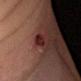biopsy_status: not biopsied; imaged during a skin examination
lighting: cross-polarized
patient:
  sex: male
  age_approx: 60
site: right forearm
image:
  source: total-body photography crop
  field_of_view_mm: 15
lesion_size:
  long_diameter_mm_approx: 3.0